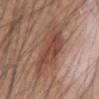<record>
<lighting>white-light</lighting>
<lesion_size>
  <long_diameter_mm_approx>8.0</long_diameter_mm_approx>
</lesion_size>
<patient>
  <sex>male</sex>
  <age_approx>60</age_approx>
</patient>
<image>
  <source>total-body photography crop</source>
  <field_of_view_mm>15</field_of_view_mm>
</image>
<site>abdomen</site>
</record>